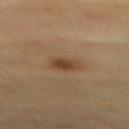acquisition: total-body-photography crop, ~15 mm field of view | lighting: cross-polarized | location: the mid back | subject: female, aged 78 to 82 | lesion size: about 3 mm | image-analysis metrics: a nevus-likeness score of about 95/100 and lesion-presence confidence of about 100/100.